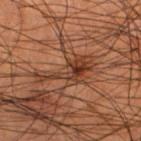Clinical summary:
Located on the leg. The subject is a male about 55 years old. A lesion tile, about 15 mm wide, cut from a 3D total-body photograph. Longest diameter approximately 6.5 mm.